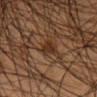A close-up tile cropped from a whole-body skin photograph, about 15 mm across. The subject is a male in their mid-40s. Approximately 2.5 mm at its widest. From the right lower leg.